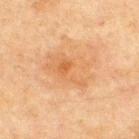<tbp_lesion>
  <biopsy_status>not biopsied; imaged during a skin examination</biopsy_status>
  <image>
    <source>total-body photography crop</source>
    <field_of_view_mm>15</field_of_view_mm>
  </image>
  <site>front of the torso</site>
  <lighting>cross-polarized</lighting>
  <lesion_size>
    <long_diameter_mm_approx>5.5</long_diameter_mm_approx>
  </lesion_size>
  <patient>
    <sex>male</sex>
    <age_approx>65</age_approx>
  </patient>
</tbp_lesion>